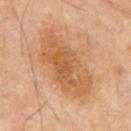Acquisition and patient details:
About 9 mm across. Captured under cross-polarized illumination. The lesion is on the mid back. The patient is a male approximately 70 years of age. This image is a 15 mm lesion crop taken from a total-body photograph. An algorithmic analysis of the crop reported a mean CIELAB color near L≈57 a*≈21 b*≈38 and a lesion-to-skin contrast of about 7 (normalized; higher = more distinct). The analysis additionally found a classifier nevus-likeness of about 0/100.